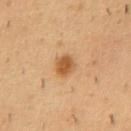notes — catalogued during a skin exam; not biopsied | patient — male, about 50 years old | location — the front of the torso | acquisition — ~15 mm tile from a whole-body skin photo.